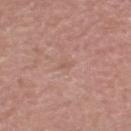workup: catalogued during a skin exam; not biopsied
location: the head or neck
subject: male, aged around 45
TBP lesion metrics: a lesion area of about 1 mm², an eccentricity of roughly 0.75, and a shape-asymmetry score of about 0.4 (0 = symmetric); border irregularity of about 3.5 on a 0–10 scale, internal color variation of about 0 on a 0–10 scale, and a peripheral color-asymmetry measure near 0
tile lighting: white-light
size: ≈1 mm
acquisition: 15 mm crop, total-body photography